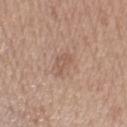Part of a total-body skin-imaging series; this lesion was reviewed on a skin check and was not flagged for biopsy.
Captured under white-light illumination.
A female patient, in their mid- to late 60s.
The total-body-photography lesion software estimated a lesion area of about 3.5 mm², a shape eccentricity near 0.85, and a shape-asymmetry score of about 0.4 (0 = symmetric). The software also gave internal color variation of about 2 on a 0–10 scale and peripheral color asymmetry of about 0.5.
About 3 mm across.
The lesion is on the left forearm.
Cropped from a whole-body photographic skin survey; the tile spans about 15 mm.